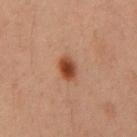No biopsy was performed on this lesion — it was imaged during a full skin examination and was not determined to be concerning. The subject is a male about 60 years old. A lesion tile, about 15 mm wide, cut from a 3D total-body photograph. From the chest.